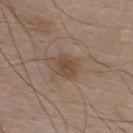Captured during whole-body skin photography for melanoma surveillance; the lesion was not biopsied. A close-up tile cropped from a whole-body skin photograph, about 15 mm across. A male subject aged around 80. Imaged with white-light lighting. On the chest.